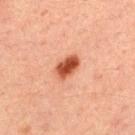Notes:
- patient — male, approximately 30 years of age
- tile lighting — cross-polarized
- acquisition — total-body-photography crop, ~15 mm field of view
- body site — the upper back